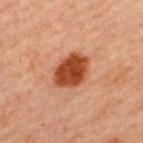<case>
  <biopsy_status>not biopsied; imaged during a skin examination</biopsy_status>
  <automated_metrics>
    <cielab_L>38</cielab_L>
    <cielab_a>26</cielab_a>
    <cielab_b>33</cielab_b>
    <vs_skin_darker_L>15.0</vs_skin_darker_L>
    <vs_skin_contrast_norm>12.5</vs_skin_contrast_norm>
    <border_irregularity_0_10>1.5</border_irregularity_0_10>
    <peripheral_color_asymmetry>1.0</peripheral_color_asymmetry>
  </automated_metrics>
  <lesion_size>
    <long_diameter_mm_approx>4.5</long_diameter_mm_approx>
  </lesion_size>
  <patient>
    <sex>male</sex>
    <age_approx>60</age_approx>
  </patient>
  <image>
    <source>total-body photography crop</source>
    <field_of_view_mm>15</field_of_view_mm>
  </image>
  <lighting>cross-polarized</lighting>
  <site>back</site>
</case>